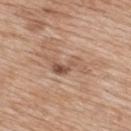location: the back
diameter: ~5.5 mm (longest diameter)
image source: ~15 mm crop, total-body skin-cancer survey
TBP lesion metrics: a shape eccentricity near 0.9 and two-axis asymmetry of about 0.6; a lesion color around L≈55 a*≈19 b*≈29 in CIELAB, about 9 CIELAB-L* units darker than the surrounding skin, and a normalized lesion–skin contrast near 6.5
subject: female, about 40 years old